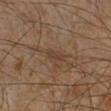– workup · imaged on a skin check; not biopsied
– automated metrics · a mean CIELAB color near L≈34 a*≈15 b*≈24 and a normalized border contrast of about 6; border irregularity of about 2.5 on a 0–10 scale, a within-lesion color-variation index near 0/10, and radial color variation of about 0; an automated nevus-likeness rating near 0 out of 100 and lesion-presence confidence of about 90/100
– site · the leg
– subject · male, in their 60s
– illumination · cross-polarized illumination
– acquisition · ~15 mm crop, total-body skin-cancer survey
– lesion diameter · ≈2.5 mm